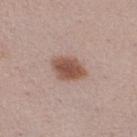acquisition=~15 mm crop, total-body skin-cancer survey; illumination=white-light; lesion diameter=about 4 mm; subject=female, aged 18 to 22; location=the right thigh.